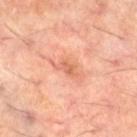notes — imaged on a skin check; not biopsied